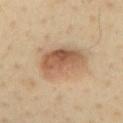Impression:
Imaged during a routine full-body skin examination; the lesion was not biopsied and no histopathology is available.
Clinical summary:
The recorded lesion diameter is about 5.5 mm. Imaged with cross-polarized lighting. A 15 mm crop from a total-body photograph taken for skin-cancer surveillance. Automated tile analysis of the lesion measured a lesion color around L≈56 a*≈18 b*≈32 in CIELAB, about 12 CIELAB-L* units darker than the surrounding skin, and a normalized border contrast of about 8.5. The analysis additionally found lesion-presence confidence of about 100/100. The patient is a male aged around 40. Located on the back.